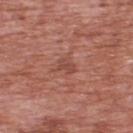lesion size: ~2.5 mm (longest diameter) | patient: male, in their 70s | site: the upper back | illumination: white-light illumination | image: ~15 mm crop, total-body skin-cancer survey | automated metrics: a lesion area of about 3.5 mm², an eccentricity of roughly 0.75, and two-axis asymmetry of about 0.25; border irregularity of about 3 on a 0–10 scale and radial color variation of about 0.5; a classifier nevus-likeness of about 0/100 and a detector confidence of about 100 out of 100 that the crop contains a lesion.